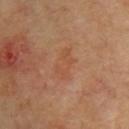pathology — a superficial basal cell carcinoma (malignant).